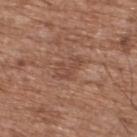Clinical impression: Captured during whole-body skin photography for melanoma surveillance; the lesion was not biopsied. Acquisition and patient details: Located on the back. A 15 mm close-up tile from a total-body photography series done for melanoma screening. The lesion's longest dimension is about 3.5 mm. A male patient, in their mid- to late 70s. Captured under white-light illumination. Automated tile analysis of the lesion measured a footprint of about 5 mm², an outline eccentricity of about 0.8 (0 = round, 1 = elongated), and two-axis asymmetry of about 0.5. The software also gave a border-irregularity rating of about 6.5/10, internal color variation of about 1.5 on a 0–10 scale, and peripheral color asymmetry of about 0.5.